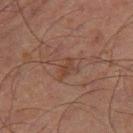tile lighting = cross-polarized | acquisition = 15 mm crop, total-body photography | location = the right thigh | subject = male, aged approximately 70 | size = ≈3.5 mm.